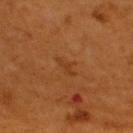follow-up=catalogued during a skin exam; not biopsied | image source=total-body-photography crop, ~15 mm field of view | site=the back | subject=male, aged approximately 60 | lesion diameter=about 2.5 mm.